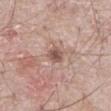Impression: This lesion was catalogued during total-body skin photography and was not selected for biopsy. Background: A male patient aged around 65. From the abdomen. A lesion tile, about 15 mm wide, cut from a 3D total-body photograph.